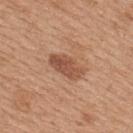The lesion was tiled from a total-body skin photograph and was not biopsied. This image is a 15 mm lesion crop taken from a total-body photograph. On the upper back. A female subject approximately 45 years of age.